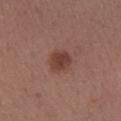Q: Was this lesion biopsied?
A: catalogued during a skin exam; not biopsied
Q: What is the anatomic site?
A: the right forearm
Q: Patient demographics?
A: female, in their 30s
Q: How was this image acquired?
A: 15 mm crop, total-body photography